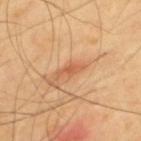No biopsy was performed on this lesion — it was imaged during a full skin examination and was not determined to be concerning. A 15 mm crop from a total-body photograph taken for skin-cancer surveillance. The lesion is located on the back. A male subject aged around 65. This is a cross-polarized tile. The lesion's longest dimension is about 3 mm. An algorithmic analysis of the crop reported a footprint of about 3 mm², an outline eccentricity of about 0.95 (0 = round, 1 = elongated), and a shape-asymmetry score of about 0.35 (0 = symmetric). The software also gave a nevus-likeness score of about 0/100.